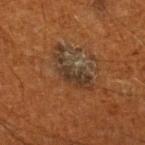Impression:
Captured during whole-body skin photography for melanoma surveillance; the lesion was not biopsied.
Context:
Longest diameter approximately 6 mm. Captured under cross-polarized illumination. A male subject, about 60 years old. The lesion is located on the right lower leg. A lesion tile, about 15 mm wide, cut from a 3D total-body photograph.